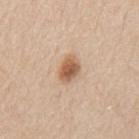Q: Is there a histopathology result?
A: total-body-photography surveillance lesion; no biopsy
Q: Illumination type?
A: white-light illumination
Q: What kind of image is this?
A: total-body-photography crop, ~15 mm field of view
Q: Lesion location?
A: the mid back
Q: Who is the patient?
A: male, aged around 60